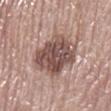Recorded during total-body skin imaging; not selected for excision or biopsy.
An algorithmic analysis of the crop reported an area of roughly 23 mm², a shape eccentricity near 0.3, and a shape-asymmetry score of about 0.1 (0 = symmetric). And it measured a lesion color around L≈50 a*≈18 b*≈22 in CIELAB, roughly 14 lightness units darker than nearby skin, and a normalized border contrast of about 10. The software also gave a nevus-likeness score of about 15/100 and lesion-presence confidence of about 100/100.
The lesion is on the right lower leg.
The recorded lesion diameter is about 5.5 mm.
Imaged with white-light lighting.
The subject is a female in their mid-60s.
A roughly 15 mm field-of-view crop from a total-body skin photograph.